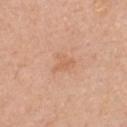workup = catalogued during a skin exam; not biopsied | patient = male, in their 50s | TBP lesion metrics = a lesion color around L≈63 a*≈22 b*≈35 in CIELAB, roughly 6 lightness units darker than nearby skin, and a normalized lesion–skin contrast near 4.5; a border-irregularity rating of about 5/10, a color-variation rating of about 1.5/10, and a peripheral color-asymmetry measure near 0.5; a nevus-likeness score of about 0/100 | site = the front of the torso | image source = 15 mm crop, total-body photography.